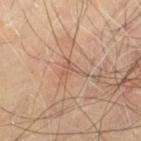The lesion was photographed on a routine skin check and not biopsied; there is no pathology result. On the leg. A male subject, approximately 70 years of age. Longest diameter approximately 3 mm. Captured under cross-polarized illumination. The total-body-photography lesion software estimated a shape eccentricity near 0.8 and a shape-asymmetry score of about 0.65 (0 = symmetric). The analysis additionally found a classifier nevus-likeness of about 0/100. A 15 mm close-up tile from a total-body photography series done for melanoma screening.